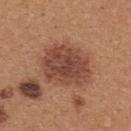workup: imaged on a skin check; not biopsied
image source: ~15 mm crop, total-body skin-cancer survey
diameter: ~6 mm (longest diameter)
site: the back
lighting: white-light
subject: female, about 40 years old